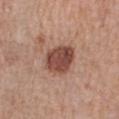{"biopsy_status": "not biopsied; imaged during a skin examination", "automated_metrics": {"area_mm2_approx": 12.0, "eccentricity": 0.6, "shape_asymmetry": 0.2, "vs_skin_darker_L": 14.0, "vs_skin_contrast_norm": 10.0}, "patient": {"sex": "female", "age_approx": 60}, "lighting": "white-light", "site": "right forearm", "lesion_size": {"long_diameter_mm_approx": 4.5}, "image": {"source": "total-body photography crop", "field_of_view_mm": 15}}